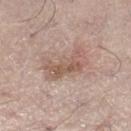{
  "biopsy_status": "not biopsied; imaged during a skin examination",
  "image": {
    "source": "total-body photography crop",
    "field_of_view_mm": 15
  },
  "lesion_size": {
    "long_diameter_mm_approx": 5.5
  },
  "patient": {
    "sex": "male",
    "age_approx": 70
  },
  "site": "right lower leg"
}